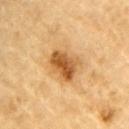Recorded during total-body skin imaging; not selected for excision or biopsy. This is a cross-polarized tile. A male subject aged around 85 or older. The total-body-photography lesion software estimated a lesion area of about 11 mm², an outline eccentricity of about 0.65 (0 = round, 1 = elongated), and a shape-asymmetry score of about 0.25 (0 = symmetric). And it measured a border-irregularity rating of about 2.5/10, a within-lesion color-variation index near 6/10, and radial color variation of about 2. A 15 mm close-up tile from a total-body photography series done for melanoma screening. The lesion's longest dimension is about 4 mm. On the left upper arm.